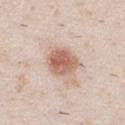Assessment: The lesion was photographed on a routine skin check and not biopsied; there is no pathology result. Clinical summary: Longest diameter approximately 4 mm. A 15 mm close-up extracted from a 3D total-body photography capture. The lesion is on the front of the torso. A male subject about 25 years old. Captured under white-light illumination.